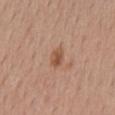Q: Was a biopsy performed?
A: no biopsy performed (imaged during a skin exam)
Q: What is the imaging modality?
A: 15 mm crop, total-body photography
Q: Patient demographics?
A: female, aged around 55
Q: What is the anatomic site?
A: the mid back
Q: Illumination type?
A: white-light illumination
Q: How large is the lesion?
A: ≈2.5 mm